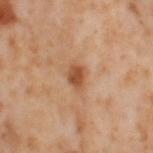The lesion was tiled from a total-body skin photograph and was not biopsied. This is a cross-polarized tile. Approximately 2.5 mm at its widest. The subject is a female aged 53–57. A 15 mm close-up extracted from a 3D total-body photography capture. From the left thigh.